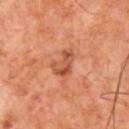  biopsy_status: not biopsied; imaged during a skin examination
  patient:
    sex: male
    age_approx: 65
  automated_metrics:
    eccentricity: 0.8
    shape_asymmetry: 0.5
  image:
    source: total-body photography crop
    field_of_view_mm: 15
  site: chest
  lighting: cross-polarized